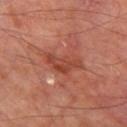Captured during whole-body skin photography for melanoma surveillance; the lesion was not biopsied.
A male subject, aged 68 to 72.
The tile uses cross-polarized illumination.
Measured at roughly 4.5 mm in maximum diameter.
The lesion is located on the left thigh.
A 15 mm crop from a total-body photograph taken for skin-cancer surveillance.